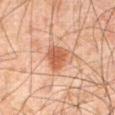Impression: Recorded during total-body skin imaging; not selected for excision or biopsy. Clinical summary: A 15 mm close-up tile from a total-body photography series done for melanoma screening. The lesion-visualizer software estimated a border-irregularity rating of about 2.5/10, internal color variation of about 2 on a 0–10 scale, and a peripheral color-asymmetry measure near 0.5. And it measured a nevus-likeness score of about 95/100 and lesion-presence confidence of about 100/100. Measured at roughly 3.5 mm in maximum diameter. Located on the abdomen. A male subject aged around 65.